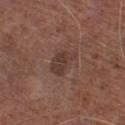Clinical impression: No biopsy was performed on this lesion — it was imaged during a full skin examination and was not determined to be concerning. Clinical summary: From the left lower leg. The tile uses white-light illumination. Cropped from a whole-body photographic skin survey; the tile spans about 15 mm. The subject is a male aged approximately 75. Approximately 4 mm at its widest.